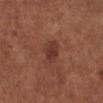Recorded during total-body skin imaging; not selected for excision or biopsy.